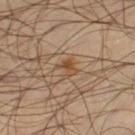Recorded during total-body skin imaging; not selected for excision or biopsy. Cropped from a whole-body photographic skin survey; the tile spans about 15 mm. A male subject aged around 45. Located on the leg. The tile uses cross-polarized illumination. The recorded lesion diameter is about 2 mm.